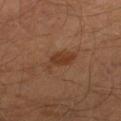Q: Is there a histopathology result?
A: total-body-photography surveillance lesion; no biopsy
Q: What is the anatomic site?
A: the right lower leg
Q: Illumination type?
A: cross-polarized
Q: Who is the patient?
A: male, in their 40s
Q: What is the lesion's diameter?
A: ~4 mm (longest diameter)
Q: How was this image acquired?
A: ~15 mm crop, total-body skin-cancer survey